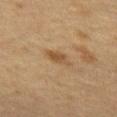Q: Where on the body is the lesion?
A: the front of the torso
Q: How large is the lesion?
A: ≈3 mm
Q: How was this image acquired?
A: 15 mm crop, total-body photography
Q: What are the patient's age and sex?
A: female, aged around 55